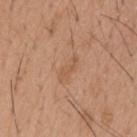biopsy status: no biopsy performed (imaged during a skin exam)
lesion size: ≈3.5 mm
location: the head or neck
patient: male, approximately 20 years of age
image source: ~15 mm crop, total-body skin-cancer survey